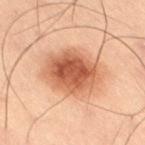Clinical impression:
The lesion was tiled from a total-body skin photograph and was not biopsied.
Background:
Approximately 5.5 mm at its widest. The lesion is on the right thigh. A male subject, aged 53–57. Imaged with cross-polarized lighting. An algorithmic analysis of the crop reported a within-lesion color-variation index near 5.5/10 and radial color variation of about 1.5. A lesion tile, about 15 mm wide, cut from a 3D total-body photograph.